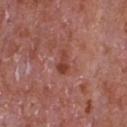workup: catalogued during a skin exam; not biopsied
patient: male, aged 63 to 67
anatomic site: the chest
automated lesion analysis: a within-lesion color-variation index near 1.5/10 and peripheral color asymmetry of about 0.5
lighting: white-light illumination
imaging modality: ~15 mm tile from a whole-body skin photo